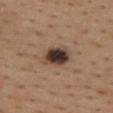Notes:
* follow-up · total-body-photography surveillance lesion; no biopsy
* site · the upper back
* image source · total-body-photography crop, ~15 mm field of view
* subject · female, roughly 40 years of age